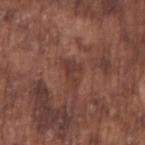Part of a total-body skin-imaging series; this lesion was reviewed on a skin check and was not flagged for biopsy.
This is a white-light tile.
Located on the right upper arm.
A male patient, aged 73 to 77.
An algorithmic analysis of the crop reported an area of roughly 4.5 mm², an outline eccentricity of about 0.75 (0 = round, 1 = elongated), and a shape-asymmetry score of about 0.55 (0 = symmetric). The analysis additionally found a border-irregularity index near 6.5/10, a within-lesion color-variation index near 1.5/10, and a peripheral color-asymmetry measure near 0.5. It also reported a classifier nevus-likeness of about 0/100 and a lesion-detection confidence of about 75/100.
A roughly 15 mm field-of-view crop from a total-body skin photograph.
About 3 mm across.